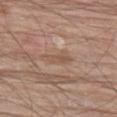{"biopsy_status": "not biopsied; imaged during a skin examination", "image": {"source": "total-body photography crop", "field_of_view_mm": 15}, "automated_metrics": {"cielab_L": 53, "cielab_a": 18, "cielab_b": 28, "vs_skin_contrast_norm": 5.0, "nevus_likeness_0_100": 0}, "site": "left thigh", "lesion_size": {"long_diameter_mm_approx": 4.0}, "patient": {"sex": "male", "age_approx": 80}}